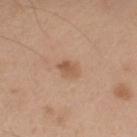Impression:
Recorded during total-body skin imaging; not selected for excision or biopsy.
Background:
The subject is a male aged 68–72. From the left upper arm. A close-up tile cropped from a whole-body skin photograph, about 15 mm across. Imaged with white-light lighting. Approximately 2.5 mm at its widest. The lesion-visualizer software estimated an average lesion color of about L≈55 a*≈21 b*≈33 (CIELAB), a lesion–skin lightness drop of about 9, and a lesion-to-skin contrast of about 6.5 (normalized; higher = more distinct).